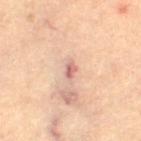<tbp_lesion>
  <biopsy_status>not biopsied; imaged during a skin examination</biopsy_status>
  <lesion_size>
    <long_diameter_mm_approx>2.5</long_diameter_mm_approx>
  </lesion_size>
  <image>
    <source>total-body photography crop</source>
    <field_of_view_mm>15</field_of_view_mm>
  </image>
  <site>left thigh</site>
  <lighting>cross-polarized</lighting>
  <automated_metrics>
    <eccentricity>0.85</eccentricity>
    <shape_asymmetry>0.25</shape_asymmetry>
    <border_irregularity_0_10>2.5</border_irregularity_0_10>
    <color_variation_0_10>1.0</color_variation_0_10>
    <peripheral_color_asymmetry>0.5</peripheral_color_asymmetry>
    <nevus_likeness_0_100>0</nevus_likeness_0_100>
  </automated_metrics>
  <patient>
    <sex>female</sex>
    <age_approx>65</age_approx>
  </patient>
</tbp_lesion>